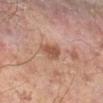image source: ~15 mm tile from a whole-body skin photo
location: the right lower leg
subject: male, about 70 years old
lesion size: about 3 mm
illumination: cross-polarized